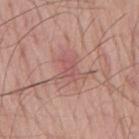Part of a total-body skin-imaging series; this lesion was reviewed on a skin check and was not flagged for biopsy. A male patient, aged 63 to 67. A close-up tile cropped from a whole-body skin photograph, about 15 mm across. The tile uses white-light illumination. Automated tile analysis of the lesion measured roughly 7 lightness units darker than nearby skin and a normalized lesion–skin contrast near 4.5. It also reported a classifier nevus-likeness of about 0/100 and a detector confidence of about 100 out of 100 that the crop contains a lesion. From the mid back.